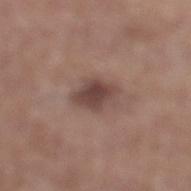{"lesion_size": {"long_diameter_mm_approx": 3.5}, "image": {"source": "total-body photography crop", "field_of_view_mm": 15}, "site": "left lower leg", "automated_metrics": {"eccentricity": 0.7, "cielab_L": 44, "cielab_a": 18, "cielab_b": 21, "vs_skin_darker_L": 11.0, "vs_skin_contrast_norm": 8.5, "color_variation_0_10": 3.5, "peripheral_color_asymmetry": 1.0, "nevus_likeness_0_100": 60, "lesion_detection_confidence_0_100": 100}, "patient": {"sex": "male", "age_approx": 65}, "lighting": "white-light"}